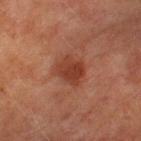Assessment: No biopsy was performed on this lesion — it was imaged during a full skin examination and was not determined to be concerning. Acquisition and patient details: Located on the arm. This is a cross-polarized tile. A lesion tile, about 15 mm wide, cut from a 3D total-body photograph. The subject is a male in their mid- to late 70s. Automated image analysis of the tile measured an automated nevus-likeness rating near 70 out of 100 and a detector confidence of about 100 out of 100 that the crop contains a lesion.